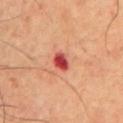Part of a total-body skin-imaging series; this lesion was reviewed on a skin check and was not flagged for biopsy.
The lesion is located on the front of the torso.
The total-body-photography lesion software estimated a mean CIELAB color near L≈48 a*≈39 b*≈31, roughly 17 lightness units darker than nearby skin, and a normalized lesion–skin contrast near 11.5. The software also gave a classifier nevus-likeness of about 0/100.
The recorded lesion diameter is about 2.5 mm.
Captured under cross-polarized illumination.
A male patient, aged around 70.
A roughly 15 mm field-of-view crop from a total-body skin photograph.